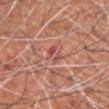Findings:
* notes: total-body-photography surveillance lesion; no biopsy
* imaging modality: total-body-photography crop, ~15 mm field of view
* location: the chest
* subject: male, approximately 60 years of age
* illumination: white-light